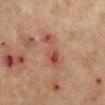Q: Is there a histopathology result?
A: total-body-photography surveillance lesion; no biopsy
Q: What is the anatomic site?
A: the left lower leg
Q: What is the lesion's diameter?
A: ≈5 mm
Q: What kind of image is this?
A: total-body-photography crop, ~15 mm field of view
Q: What did automated image analysis measure?
A: an area of roughly 10 mm², a shape eccentricity near 0.9, and a shape-asymmetry score of about 0.35 (0 = symmetric); a mean CIELAB color near L≈52 a*≈26 b*≈29, a lesion–skin lightness drop of about 9, and a lesion-to-skin contrast of about 6.5 (normalized; higher = more distinct); a classifier nevus-likeness of about 0/100 and lesion-presence confidence of about 100/100
Q: Patient demographics?
A: male, approximately 65 years of age